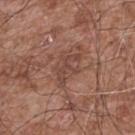Findings:
– acquisition — 15 mm crop, total-body photography
– anatomic site — the upper back
– image-analysis metrics — an automated nevus-likeness rating near 0 out of 100
– subject — male, roughly 55 years of age
– lesion size — ~4 mm (longest diameter)